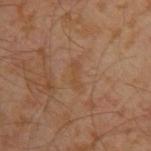No biopsy was performed on this lesion — it was imaged during a full skin examination and was not determined to be concerning. Cropped from a total-body skin-imaging series; the visible field is about 15 mm. Approximately 3 mm at its widest. Automated image analysis of the tile measured a footprint of about 3 mm², an eccentricity of roughly 0.95, and a symmetry-axis asymmetry near 0.25. The software also gave a mean CIELAB color near L≈45 a*≈19 b*≈33 and a lesion-to-skin contrast of about 4.5 (normalized; higher = more distinct). It also reported border irregularity of about 3.5 on a 0–10 scale and internal color variation of about 0 on a 0–10 scale. From the left upper arm. The patient is a male approximately 30 years of age.